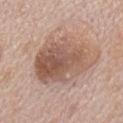• biopsy status: imaged on a skin check; not biopsied
• lesion size: about 7.5 mm
• imaging modality: 15 mm crop, total-body photography
• anatomic site: the mid back
• subject: male, aged 68 to 72